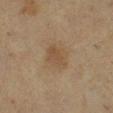| field | value |
|---|---|
| biopsy status | total-body-photography surveillance lesion; no biopsy |
| anatomic site | the right lower leg |
| subject | female, in their mid- to late 50s |
| illumination | cross-polarized illumination |
| image source | total-body-photography crop, ~15 mm field of view |